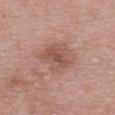Assessment: This lesion was catalogued during total-body skin photography and was not selected for biopsy. Clinical summary: A female patient aged around 50. The lesion is located on the chest. This image is a 15 mm lesion crop taken from a total-body photograph.